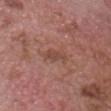Assessment: The lesion was photographed on a routine skin check and not biopsied; there is no pathology result. Acquisition and patient details: Captured under white-light illumination. A male patient, about 75 years old. Cropped from a whole-body photographic skin survey; the tile spans about 15 mm. From the chest. Approximately 3.5 mm at its widest.